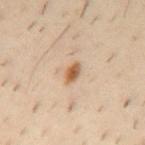biopsy_status: not biopsied; imaged during a skin examination
automated_metrics:
  border_irregularity_0_10: 2.5
  color_variation_0_10: 5.0
  peripheral_color_asymmetry: 2.0
  lesion_detection_confidence_0_100: 100
site: mid back
image:
  source: total-body photography crop
  field_of_view_mm: 15
lesion_size:
  long_diameter_mm_approx: 3.0
patient:
  sex: male
  age_approx: 40
lighting: cross-polarized